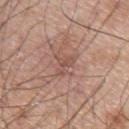Part of a total-body skin-imaging series; this lesion was reviewed on a skin check and was not flagged for biopsy. Automated image analysis of the tile measured a lesion color around L≈52 a*≈21 b*≈25 in CIELAB and a normalized lesion–skin contrast near 5. And it measured a classifier nevus-likeness of about 0/100 and a lesion-detection confidence of about 95/100. The lesion is located on the arm. A region of skin cropped from a whole-body photographic capture, roughly 15 mm wide. The tile uses white-light illumination. The subject is a male aged 63 to 67. The recorded lesion diameter is about 2.5 mm.